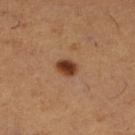subject=female, aged approximately 55 | body site=the right lower leg | illumination=cross-polarized | TBP lesion metrics=an eccentricity of roughly 0.65 and a symmetry-axis asymmetry near 0.2; a lesion color around L≈40 a*≈23 b*≈33 in CIELAB and roughly 15 lightness units darker than nearby skin; a border-irregularity index near 1.5/10 and radial color variation of about 1 | acquisition=~15 mm tile from a whole-body skin photo | lesion diameter=~2.5 mm (longest diameter).